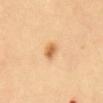  biopsy_status: not biopsied; imaged during a skin examination
  patient:
    sex: female
    age_approx: 35
  image:
    source: total-body photography crop
    field_of_view_mm: 15
  site: abdomen
  lesion_size:
    long_diameter_mm_approx: 2.5
  automated_metrics:
    cielab_L: 64
    cielab_a: 22
    cielab_b: 42
    vs_skin_darker_L: 12.0
    border_irregularity_0_10: 2.0
    color_variation_0_10: 2.0
    peripheral_color_asymmetry: 1.0
    nevus_likeness_0_100: 95
    lesion_detection_confidence_0_100: 100